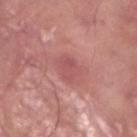follow-up = no biopsy performed (imaged during a skin exam)
subject = male, aged 38–42
anatomic site = the left lower leg
illumination = white-light
image source = 15 mm crop, total-body photography